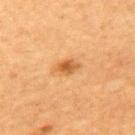follow-up: imaged on a skin check; not biopsied | TBP lesion metrics: a lesion–skin lightness drop of about 10 and a lesion-to-skin contrast of about 7.5 (normalized; higher = more distinct); internal color variation of about 3.5 on a 0–10 scale and a peripheral color-asymmetry measure near 1 | subject: female, aged approximately 60 | image source: total-body-photography crop, ~15 mm field of view | body site: the upper back | lesion diameter: ≈2.5 mm.